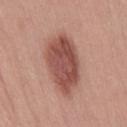Imaged during a routine full-body skin examination; the lesion was not biopsied and no histopathology is available. The tile uses white-light illumination. About 8 mm across. A female patient aged 28–32. Cropped from a total-body skin-imaging series; the visible field is about 15 mm. The lesion is located on the right thigh. Automated tile analysis of the lesion measured a border-irregularity rating of about 2.5/10, internal color variation of about 4 on a 0–10 scale, and a peripheral color-asymmetry measure near 1.5. And it measured an automated nevus-likeness rating near 90 out of 100.